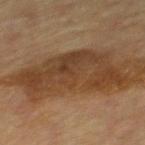The lesion was photographed on a routine skin check and not biopsied; there is no pathology result.
Measured at roughly 10.5 mm in maximum diameter.
The patient is a male aged 63 to 67.
A region of skin cropped from a whole-body photographic capture, roughly 15 mm wide.
The lesion is located on the mid back.
This is a cross-polarized tile.